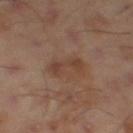diameter — about 4.5 mm
location — the right thigh
image — total-body-photography crop, ~15 mm field of view
lighting — cross-polarized illumination
patient — male, roughly 45 years of age